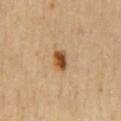No biopsy was performed on this lesion — it was imaged during a full skin examination and was not determined to be concerning.
From the mid back.
The tile uses cross-polarized illumination.
A close-up tile cropped from a whole-body skin photograph, about 15 mm across.
The patient is a female in their mid-50s.
Approximately 3 mm at its widest.